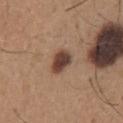biopsy_status: not biopsied; imaged during a skin examination
site: chest
image:
  source: total-body photography crop
  field_of_view_mm: 15
patient:
  sex: male
  age_approx: 65
automated_metrics:
  area_mm2_approx: 6.5
  eccentricity: 0.75
  color_variation_0_10: 4.0
  peripheral_color_asymmetry: 1.0
lighting: white-light